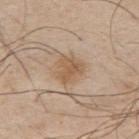Case summary:
– workup · imaged on a skin check; not biopsied
– TBP lesion metrics · a border-irregularity rating of about 3/10 and peripheral color asymmetry of about 1; a nevus-likeness score of about 65/100 and a lesion-detection confidence of about 100/100
– lesion diameter · ~3.5 mm (longest diameter)
– illumination · white-light
– anatomic site · the upper back
– acquisition · ~15 mm crop, total-body skin-cancer survey
– patient · male, approximately 30 years of age